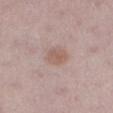Q: Was this lesion biopsied?
A: total-body-photography surveillance lesion; no biopsy
Q: Illumination type?
A: white-light illumination
Q: Lesion size?
A: about 2.5 mm
Q: What are the patient's age and sex?
A: female, roughly 30 years of age
Q: Where on the body is the lesion?
A: the left lower leg
Q: Automated lesion metrics?
A: a footprint of about 4.5 mm² and an eccentricity of roughly 0.65; an average lesion color of about L≈57 a*≈18 b*≈24 (CIELAB) and a normalized lesion–skin contrast near 6; a color-variation rating of about 2/10 and radial color variation of about 0.5; a lesion-detection confidence of about 100/100
Q: What kind of image is this?
A: total-body-photography crop, ~15 mm field of view